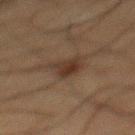Recorded during total-body skin imaging; not selected for excision or biopsy.
A roughly 15 mm field-of-view crop from a total-body skin photograph.
The lesion is located on the mid back.
Approximately 3 mm at its widest.
A male patient about 60 years old.
Captured under cross-polarized illumination.